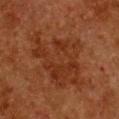subject=female, roughly 50 years of age | location=the chest | illumination=cross-polarized | size=about 8.5 mm | image source=~15 mm tile from a whole-body skin photo.